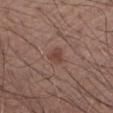The lesion is on the right upper arm.
A male patient, aged approximately 55.
A 15 mm crop from a total-body photograph taken for skin-cancer surveillance.
The recorded lesion diameter is about 2.5 mm.
Captured under white-light illumination.
An algorithmic analysis of the crop reported a footprint of about 3 mm² and a symmetry-axis asymmetry near 0.45. And it measured a border-irregularity rating of about 4/10 and a color-variation rating of about 1.5/10.